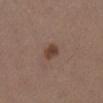Assessment:
The lesion was photographed on a routine skin check and not biopsied; there is no pathology result.
Context:
The tile uses white-light illumination. A 15 mm close-up tile from a total-body photography series done for melanoma screening. Approximately 2.5 mm at its widest. Located on the right lower leg. A female subject aged around 40.